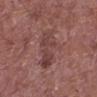This lesion was catalogued during total-body skin photography and was not selected for biopsy. A male subject, aged 63–67. A 15 mm close-up tile from a total-body photography series done for melanoma screening. The lesion is on the left lower leg.